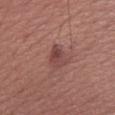Q: Was this lesion biopsied?
A: total-body-photography surveillance lesion; no biopsy
Q: What is the imaging modality?
A: ~15 mm tile from a whole-body skin photo
Q: What is the anatomic site?
A: the left forearm
Q: What are the patient's age and sex?
A: male, in their mid-30s